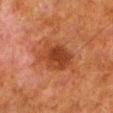workup — imaged on a skin check; not biopsied
anatomic site — the left lower leg
automated lesion analysis — a shape eccentricity near 0.75 and a shape-asymmetry score of about 0.2 (0 = symmetric); a classifier nevus-likeness of about 70/100 and lesion-presence confidence of about 100/100
illumination — cross-polarized illumination
patient — male, approximately 80 years of age
imaging modality — total-body-photography crop, ~15 mm field of view
size — ~5 mm (longest diameter)